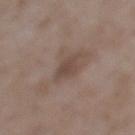The lesion was photographed on a routine skin check and not biopsied; there is no pathology result. A 15 mm close-up extracted from a 3D total-body photography capture. The recorded lesion diameter is about 2.5 mm. A female patient roughly 70 years of age. The lesion is on the upper back. The tile uses white-light illumination.